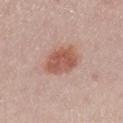Clinical summary: Automated tile analysis of the lesion measured a mean CIELAB color near L≈55 a*≈24 b*≈28, about 12 CIELAB-L* units darker than the surrounding skin, and a normalized border contrast of about 8.5. It also reported a border-irregularity index near 2/10 and internal color variation of about 3.5 on a 0–10 scale. Located on the leg. A female subject, aged 23–27. Captured under white-light illumination. The recorded lesion diameter is about 4.5 mm. A 15 mm close-up tile from a total-body photography series done for melanoma screening.